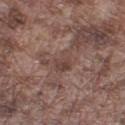anatomic site: the leg
subject: male, about 75 years old
lesion size: ≈3.5 mm
tile lighting: white-light illumination
automated metrics: an area of roughly 5.5 mm², an eccentricity of roughly 0.8, and two-axis asymmetry of about 0.3; border irregularity of about 3 on a 0–10 scale and a within-lesion color-variation index near 4.5/10
image source: ~15 mm tile from a whole-body skin photo